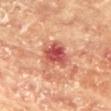The lesion was tiled from a total-body skin photograph and was not biopsied. The tile uses cross-polarized illumination. A lesion tile, about 15 mm wide, cut from a 3D total-body photograph. Located on the chest. Automated tile analysis of the lesion measured an area of roughly 12 mm² and a shape-asymmetry score of about 0.25 (0 = symmetric). The software also gave an average lesion color of about L≈51 a*≈30 b*≈28 (CIELAB) and a normalized lesion–skin contrast near 9. It also reported a detector confidence of about 100 out of 100 that the crop contains a lesion. A female subject, roughly 80 years of age.